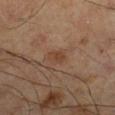The lesion was photographed on a routine skin check and not biopsied; there is no pathology result. A male patient, about 60 years old. This is a cross-polarized tile. From the left lower leg. A roughly 15 mm field-of-view crop from a total-body skin photograph.